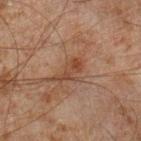* biopsy status — no biopsy performed (imaged during a skin exam)
* image — ~15 mm tile from a whole-body skin photo
* illumination — cross-polarized
* subject — male, roughly 45 years of age
* anatomic site — the right lower leg
* size — ≈3.5 mm